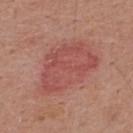Part of a total-body skin-imaging series; this lesion was reviewed on a skin check and was not flagged for biopsy.
A 15 mm close-up tile from a total-body photography series done for melanoma screening.
The lesion is located on the upper back.
A male subject in their mid- to late 50s.